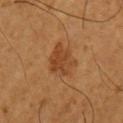workup=no biopsy performed (imaged during a skin exam) | subject=male, about 65 years old | lesion size=≈4.5 mm | tile lighting=cross-polarized illumination | body site=the left upper arm | image=~15 mm tile from a whole-body skin photo.